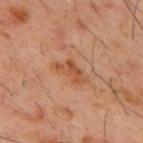- follow-up — catalogued during a skin exam; not biopsied
- subject — male, approximately 60 years of age
- image — total-body-photography crop, ~15 mm field of view
- location — the mid back
- lesion diameter — ≈4 mm
- lighting — cross-polarized illumination